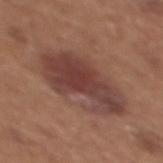Q: Was a biopsy performed?
A: imaged on a skin check; not biopsied
Q: What kind of image is this?
A: ~15 mm crop, total-body skin-cancer survey
Q: How large is the lesion?
A: ≈8.5 mm
Q: Lesion location?
A: the chest
Q: How was the tile lit?
A: white-light illumination
Q: What are the patient's age and sex?
A: female, approximately 35 years of age